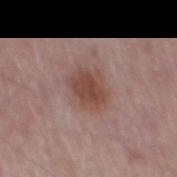Recorded during total-body skin imaging; not selected for excision or biopsy.
Cropped from a whole-body photographic skin survey; the tile spans about 15 mm.
The patient is a male in their mid- to late 60s.
The lesion is on the mid back.
The lesion's longest dimension is about 4 mm.